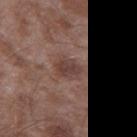  biopsy_status: not biopsied; imaged during a skin examination
  patient:
    sex: male
    age_approx: 45
  image:
    source: total-body photography crop
    field_of_view_mm: 15
  site: left thigh
  lighting: white-light